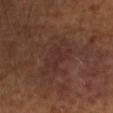– biopsy status — total-body-photography surveillance lesion; no biopsy
– size — about 5 mm
– imaging modality — 15 mm crop, total-body photography
– site — the arm
– subject — male, aged 53 to 57
– TBP lesion metrics — an area of roughly 10 mm² and a symmetry-axis asymmetry near 0.35; a lesion color around L≈27 a*≈18 b*≈20 in CIELAB and about 4 CIELAB-L* units darker than the surrounding skin; a within-lesion color-variation index near 2/10 and radial color variation of about 0.5; a nevus-likeness score of about 0/100 and a detector confidence of about 100 out of 100 that the crop contains a lesion
– tile lighting — cross-polarized